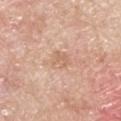Imaged during a routine full-body skin examination; the lesion was not biopsied and no histopathology is available. Captured under white-light illumination. Located on the upper back. The subject is a male aged around 65. Cropped from a total-body skin-imaging series; the visible field is about 15 mm.